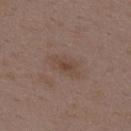| key | value |
|---|---|
| notes | total-body-photography surveillance lesion; no biopsy |